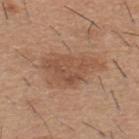This lesion was catalogued during total-body skin photography and was not selected for biopsy. The lesion's longest dimension is about 7 mm. A male patient, aged approximately 60. The lesion-visualizer software estimated a footprint of about 19 mm², an eccentricity of roughly 0.8, and two-axis asymmetry of about 0.35. The software also gave border irregularity of about 4.5 on a 0–10 scale, internal color variation of about 3.5 on a 0–10 scale, and a peripheral color-asymmetry measure near 1. From the back. A 15 mm close-up tile from a total-body photography series done for melanoma screening. Captured under white-light illumination.